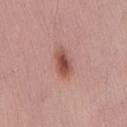Part of a total-body skin-imaging series; this lesion was reviewed on a skin check and was not flagged for biopsy. Located on the lower back. A male patient, aged approximately 30. A close-up tile cropped from a whole-body skin photograph, about 15 mm across.